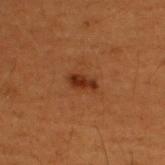The lesion was tiled from a total-body skin photograph and was not biopsied. Approximately 3 mm at its widest. A male patient about 50 years old. From the upper back. This is a cross-polarized tile. Automated image analysis of the tile measured a lesion color around L≈25 a*≈21 b*≈28 in CIELAB, roughly 9 lightness units darker than nearby skin, and a normalized border contrast of about 9.5. And it measured a border-irregularity index near 3/10, a color-variation rating of about 1.5/10, and peripheral color asymmetry of about 0.5. The analysis additionally found a classifier nevus-likeness of about 90/100. A 15 mm crop from a total-body photograph taken for skin-cancer surveillance.